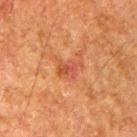{
  "biopsy_status": "not biopsied; imaged during a skin examination",
  "patient": {
    "sex": "male",
    "age_approx": 80
  },
  "lesion_size": {
    "long_diameter_mm_approx": 2.5
  },
  "lighting": "cross-polarized",
  "automated_metrics": {
    "area_mm2_approx": 3.5,
    "shape_asymmetry": 0.3,
    "border_irregularity_0_10": 3.5,
    "color_variation_0_10": 3.5,
    "nevus_likeness_0_100": 0,
    "lesion_detection_confidence_0_100": 100
  },
  "site": "right upper arm",
  "image": {
    "source": "total-body photography crop",
    "field_of_view_mm": 15
  }
}